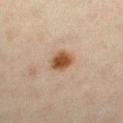<record>
  <biopsy_status>not biopsied; imaged during a skin examination</biopsy_status>
  <patient>
    <sex>female</sex>
    <age_approx>45</age_approx>
  </patient>
  <image>
    <source>total-body photography crop</source>
    <field_of_view_mm>15</field_of_view_mm>
  </image>
  <site>right lower leg</site>
  <lesion_size>
    <long_diameter_mm_approx>3.0</long_diameter_mm_approx>
  </lesion_size>
</record>